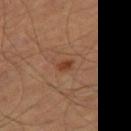• workup: imaged on a skin check; not biopsied
• subject: male, in their mid- to late 60s
• body site: the leg
• imaging modality: 15 mm crop, total-body photography
• lesion size: ≈2 mm